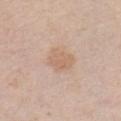Clinical impression: The lesion was photographed on a routine skin check and not biopsied; there is no pathology result. Acquisition and patient details: A 15 mm close-up extracted from a 3D total-body photography capture. On the chest. A female subject aged 33 to 37.